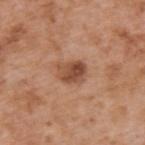Case summary:
- notes: catalogued during a skin exam; not biopsied
- lighting: white-light illumination
- image-analysis metrics: a mean CIELAB color near L≈49 a*≈23 b*≈32
- subject: male, aged 63–67
- anatomic site: the upper back
- image: 15 mm crop, total-body photography